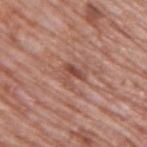<record>
<biopsy_status>not biopsied; imaged during a skin examination</biopsy_status>
<image>
  <source>total-body photography crop</source>
  <field_of_view_mm>15</field_of_view_mm>
</image>
<lesion_size>
  <long_diameter_mm_approx>2.5</long_diameter_mm_approx>
</lesion_size>
<site>upper back</site>
<lighting>white-light</lighting>
<patient>
  <sex>male</sex>
  <age_approx>70</age_approx>
</patient>
<automated_metrics>
  <area_mm2_approx>4.0</area_mm2_approx>
  <eccentricity>0.65</eccentricity>
  <shape_asymmetry>0.65</shape_asymmetry>
  <cielab_L>48</cielab_L>
  <cielab_a>24</cielab_a>
  <cielab_b>27</cielab_b>
  <vs_skin_contrast_norm>7.0</vs_skin_contrast_norm>
  <nevus_likeness_0_100>0</nevus_likeness_0_100>
</automated_metrics>
</record>